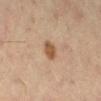biopsy status: no biopsy performed (imaged during a skin exam)
automated lesion analysis: a footprint of about 4 mm², a shape eccentricity near 0.6, and two-axis asymmetry of about 0.25; a lesion color around L≈51 a*≈19 b*≈33 in CIELAB, a lesion–skin lightness drop of about 12, and a normalized lesion–skin contrast near 9; internal color variation of about 1 on a 0–10 scale and peripheral color asymmetry of about 0.5
lesion size: ≈2.5 mm
patient: female, aged approximately 65
tile lighting: cross-polarized illumination
image: ~15 mm tile from a whole-body skin photo
location: the left leg